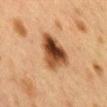• follow-up · catalogued during a skin exam; not biopsied
• patient · female, about 40 years old
• site · the back
• image · ~15 mm tile from a whole-body skin photo
• lesion diameter · about 5.5 mm